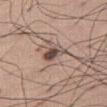Assessment: The lesion was tiled from a total-body skin photograph and was not biopsied. Clinical summary: Automated image analysis of the tile measured a mean CIELAB color near L≈48 a*≈16 b*≈21, roughly 15 lightness units darker than nearby skin, and a lesion-to-skin contrast of about 10.5 (normalized; higher = more distinct). The analysis additionally found a classifier nevus-likeness of about 30/100 and lesion-presence confidence of about 90/100. Captured under white-light illumination. The subject is a male in their mid- to late 60s. From the right thigh. A roughly 15 mm field-of-view crop from a total-body skin photograph. Longest diameter approximately 4 mm.